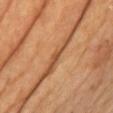The lesion was photographed on a routine skin check and not biopsied; there is no pathology result.
A lesion tile, about 15 mm wide, cut from a 3D total-body photograph.
The patient is a female in their mid-60s.
About 1.5 mm across.
Imaged with cross-polarized lighting.
The lesion is located on the chest.
Automated tile analysis of the lesion measured a footprint of about 1 mm², a shape eccentricity near 0.75, and two-axis asymmetry of about 0.5. It also reported an average lesion color of about L≈50 a*≈25 b*≈38 (CIELAB), about 10 CIELAB-L* units darker than the surrounding skin, and a normalized lesion–skin contrast near 7.5. And it measured a border-irregularity index near 3.5/10. The software also gave an automated nevus-likeness rating near 0 out of 100 and a lesion-detection confidence of about 0/100.